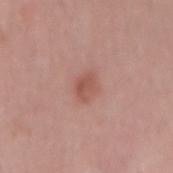Imaged during a routine full-body skin examination; the lesion was not biopsied and no histopathology is available. Longest diameter approximately 3 mm. Captured under white-light illumination. Automated image analysis of the tile measured an area of roughly 5 mm², an eccentricity of roughly 0.7, and two-axis asymmetry of about 0.25. The lesion is located on the mid back. A lesion tile, about 15 mm wide, cut from a 3D total-body photograph. The patient is a male roughly 50 years of age.